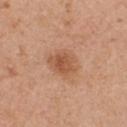- follow-up — catalogued during a skin exam; not biopsied
- acquisition — total-body-photography crop, ~15 mm field of view
- illumination — white-light illumination
- lesion size — ≈4 mm
- patient — female, in their mid- to late 40s
- location — the chest
- automated lesion analysis — a lesion area of about 9 mm², an eccentricity of roughly 0.55, and two-axis asymmetry of about 0.2; a classifier nevus-likeness of about 70/100